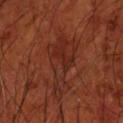{"biopsy_status": "not biopsied; imaged during a skin examination", "image": {"source": "total-body photography crop", "field_of_view_mm": 15}, "site": "front of the torso", "lesion_size": {"long_diameter_mm_approx": 8.0}, "lighting": "cross-polarized", "patient": {"sex": "male", "age_approx": 70}}